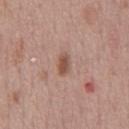A male subject aged 43–47.
The lesion is located on the chest.
The tile uses white-light illumination.
Automated tile analysis of the lesion measured an area of roughly 4 mm², an outline eccentricity of about 0.85 (0 = round, 1 = elongated), and a symmetry-axis asymmetry near 0.35.
Cropped from a whole-body photographic skin survey; the tile spans about 15 mm.
The recorded lesion diameter is about 3 mm.